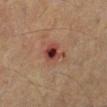Clinical impression:
No biopsy was performed on this lesion — it was imaged during a full skin examination and was not determined to be concerning.
Background:
The patient is a male roughly 55 years of age. From the left lower leg. A 15 mm close-up extracted from a 3D total-body photography capture.